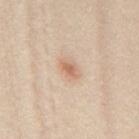Clinical impression: Captured during whole-body skin photography for melanoma surveillance; the lesion was not biopsied. Context: A 15 mm crop from a total-body photograph taken for skin-cancer surveillance. The subject is a female aged approximately 20. The lesion is on the mid back.